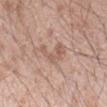Captured during whole-body skin photography for melanoma surveillance; the lesion was not biopsied.
Approximately 4 mm at its widest.
The lesion is located on the right forearm.
The total-body-photography lesion software estimated a lesion color around L≈58 a*≈19 b*≈27 in CIELAB, roughly 8 lightness units darker than nearby skin, and a normalized border contrast of about 5.5. The software also gave a border-irregularity rating of about 7/10 and a peripheral color-asymmetry measure near 1. The analysis additionally found a classifier nevus-likeness of about 0/100 and a lesion-detection confidence of about 100/100.
A close-up tile cropped from a whole-body skin photograph, about 15 mm across.
The patient is a male aged 23 to 27.
This is a white-light tile.